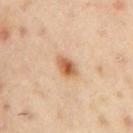| feature | finding |
|---|---|
| workup | total-body-photography surveillance lesion; no biopsy |
| image source | 15 mm crop, total-body photography |
| body site | the right upper arm |
| tile lighting | cross-polarized illumination |
| subject | male, in their mid- to late 50s |
| lesion diameter | about 3 mm |